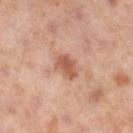Assessment:
Part of a total-body skin-imaging series; this lesion was reviewed on a skin check and was not flagged for biopsy.